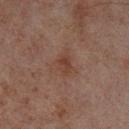Impression: Part of a total-body skin-imaging series; this lesion was reviewed on a skin check and was not flagged for biopsy. Background: The recorded lesion diameter is about 2.5 mm. From the left lower leg. Automated image analysis of the tile measured a footprint of about 4 mm² and a shape eccentricity near 0.75. Cropped from a total-body skin-imaging series; the visible field is about 15 mm. A male patient aged 68 to 72.